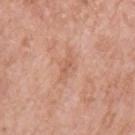{
  "biopsy_status": "not biopsied; imaged during a skin examination",
  "lesion_size": {
    "long_diameter_mm_approx": 3.0
  },
  "site": "left upper arm",
  "image": {
    "source": "total-body photography crop",
    "field_of_view_mm": 15
  },
  "patient": {
    "sex": "female",
    "age_approx": 60
  }
}